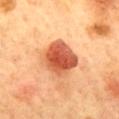<record>
  <biopsy_status>not biopsied; imaged during a skin examination</biopsy_status>
  <lesion_size>
    <long_diameter_mm_approx>4.5</long_diameter_mm_approx>
  </lesion_size>
  <lighting>cross-polarized</lighting>
  <patient>
    <sex>female</sex>
    <age_approx>60</age_approx>
  </patient>
  <site>mid back</site>
  <image>
    <source>total-body photography crop</source>
    <field_of_view_mm>15</field_of_view_mm>
  </image>
</record>